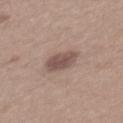The lesion was tiled from a total-body skin photograph and was not biopsied. A male subject, about 55 years old. The tile uses white-light illumination. About 3.5 mm across. A lesion tile, about 15 mm wide, cut from a 3D total-body photograph. From the back.